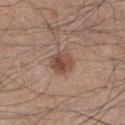follow-up=no biopsy performed (imaged during a skin exam)
image=~15 mm crop, total-body skin-cancer survey
size=≈3.5 mm
lighting=white-light
location=the left lower leg
patient=male, approximately 45 years of age